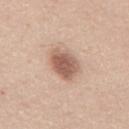Q: Was this lesion biopsied?
A: catalogued during a skin exam; not biopsied
Q: What kind of image is this?
A: ~15 mm crop, total-body skin-cancer survey
Q: Patient demographics?
A: male, approximately 50 years of age
Q: Lesion location?
A: the mid back
Q: Lesion size?
A: ~4 mm (longest diameter)